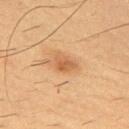  biopsy_status: not biopsied; imaged during a skin examination
  patient:
    sex: male
    age_approx: 55
  lesion_size:
    long_diameter_mm_approx: 2.5
  site: left upper arm
  lighting: cross-polarized
  image:
    source: total-body photography crop
    field_of_view_mm: 15